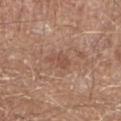The lesion was photographed on a routine skin check and not biopsied; there is no pathology result. This is a white-light tile. A male patient about 65 years old. The lesion is located on the leg. A close-up tile cropped from a whole-body skin photograph, about 15 mm across. Measured at roughly 2.5 mm in maximum diameter.